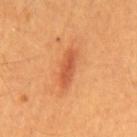| key | value |
|---|---|
| workup | imaged on a skin check; not biopsied |
| patient | male, in their 60s |
| tile lighting | cross-polarized |
| image-analysis metrics | a footprint of about 6.5 mm², an eccentricity of roughly 0.95, and two-axis asymmetry of about 0.15; an average lesion color of about L≈51 a*≈29 b*≈38 (CIELAB), about 10 CIELAB-L* units darker than the surrounding skin, and a lesion-to-skin contrast of about 7 (normalized; higher = more distinct) |
| site | the mid back |
| diameter | ~4.5 mm (longest diameter) |
| imaging modality | total-body-photography crop, ~15 mm field of view |